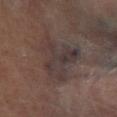This lesion was catalogued during total-body skin photography and was not selected for biopsy. A 15 mm close-up extracted from a 3D total-body photography capture. On the right lower leg. This is a cross-polarized tile. About 6 mm across. The subject is a male in their mid- to late 60s.